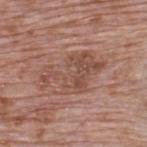Clinical impression:
Recorded during total-body skin imaging; not selected for excision or biopsy.
Background:
A close-up tile cropped from a whole-body skin photograph, about 15 mm across. A male subject aged around 70. The recorded lesion diameter is about 7 mm. Located on the upper back.